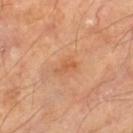<lesion>
<biopsy_status>not biopsied; imaged during a skin examination</biopsy_status>
<lighting>cross-polarized</lighting>
<image>
  <source>total-body photography crop</source>
  <field_of_view_mm>15</field_of_view_mm>
</image>
<patient>
  <sex>male</sex>
  <age_approx>70</age_approx>
</patient>
<automated_metrics>
  <eccentricity>0.9</eccentricity>
  <shape_asymmetry>0.35</shape_asymmetry>
  <vs_skin_darker_L>7.0</vs_skin_darker_L>
  <vs_skin_contrast_norm>5.5</vs_skin_contrast_norm>
  <border_irregularity_0_10>4.0</border_irregularity_0_10>
  <color_variation_0_10>0.0</color_variation_0_10>
</automated_metrics>
<lesion_size>
  <long_diameter_mm_approx>2.5</long_diameter_mm_approx>
</lesion_size>
<site>left thigh</site>
</lesion>